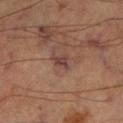Case summary:
– follow-up — no biopsy performed (imaged during a skin exam)
– lighting — cross-polarized
– acquisition — ~15 mm crop, total-body skin-cancer survey
– patient — male, aged 63 to 67
– diameter — ≈2.5 mm
– site — the right lower leg